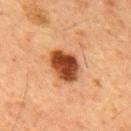This lesion was catalogued during total-body skin photography and was not selected for biopsy.
Captured under cross-polarized illumination.
The lesion's longest dimension is about 4.5 mm.
Cropped from a total-body skin-imaging series; the visible field is about 15 mm.
Automated tile analysis of the lesion measured a border-irregularity index near 2/10 and a color-variation rating of about 6/10. The analysis additionally found a nevus-likeness score of about 100/100.
A male subject approximately 60 years of age.